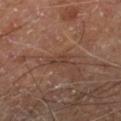| feature | finding |
|---|---|
| workup | catalogued during a skin exam; not biopsied |
| size | ~2.5 mm (longest diameter) |
| patient | male, aged 68 to 72 |
| automated metrics | a lesion area of about 3 mm² and two-axis asymmetry of about 0.4; a border-irregularity rating of about 4/10, a color-variation rating of about 1.5/10, and a peripheral color-asymmetry measure near 0.5 |
| image | total-body-photography crop, ~15 mm field of view |
| lighting | cross-polarized illumination |
| body site | the leg |